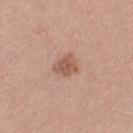Measured at roughly 3 mm in maximum diameter. A female patient roughly 55 years of age. The total-body-photography lesion software estimated a lesion area of about 5 mm² and an eccentricity of roughly 0.55. On the right thigh. A region of skin cropped from a whole-body photographic capture, roughly 15 mm wide.